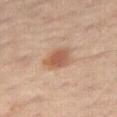Q: Was this lesion biopsied?
A: total-body-photography surveillance lesion; no biopsy
Q: What lighting was used for the tile?
A: cross-polarized illumination
Q: What is the lesion's diameter?
A: ~4 mm (longest diameter)
Q: Patient demographics?
A: male, aged approximately 60
Q: How was this image acquired?
A: 15 mm crop, total-body photography
Q: What is the anatomic site?
A: the right thigh